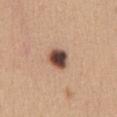follow-up: catalogued during a skin exam; not biopsied
site: the chest
subject: female, aged 28 to 32
image-analysis metrics: a footprint of about 6 mm², a shape eccentricity near 0.55, and a shape-asymmetry score of about 0.25 (0 = symmetric); a border-irregularity index near 2/10
acquisition: 15 mm crop, total-body photography
illumination: white-light